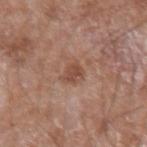{"biopsy_status": "not biopsied; imaged during a skin examination", "lesion_size": {"long_diameter_mm_approx": 2.5}, "site": "left upper arm", "lighting": "white-light", "image": {"source": "total-body photography crop", "field_of_view_mm": 15}, "patient": {"sex": "male", "age_approx": 60}, "automated_metrics": {"area_mm2_approx": 4.0, "eccentricity": 0.6, "shape_asymmetry": 0.25, "cielab_L": 48, "cielab_a": 22, "cielab_b": 28, "vs_skin_darker_L": 9.0, "vs_skin_contrast_norm": 7.0, "nevus_likeness_0_100": 35, "lesion_detection_confidence_0_100": 100}}